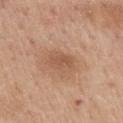diameter = about 3 mm | site = the back | subject = female, aged 63 to 67 | acquisition = ~15 mm tile from a whole-body skin photo.